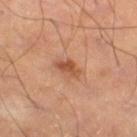Q: Was this lesion biopsied?
A: total-body-photography surveillance lesion; no biopsy
Q: What is the lesion's diameter?
A: about 3 mm
Q: How was the tile lit?
A: cross-polarized
Q: How was this image acquired?
A: 15 mm crop, total-body photography
Q: What did automated image analysis measure?
A: about 10 CIELAB-L* units darker than the surrounding skin and a normalized lesion–skin contrast near 8; a border-irregularity index near 4.5/10, a within-lesion color-variation index near 1/10, and a peripheral color-asymmetry measure near 0.5; a nevus-likeness score of about 70/100 and a lesion-detection confidence of about 100/100
Q: Lesion location?
A: the right thigh
Q: Who is the patient?
A: male, in their mid-50s